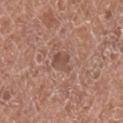<record>
<biopsy_status>not biopsied; imaged during a skin examination</biopsy_status>
<image>
  <source>total-body photography crop</source>
  <field_of_view_mm>15</field_of_view_mm>
</image>
<lighting>white-light</lighting>
<patient>
  <sex>female</sex>
  <age_approx>50</age_approx>
</patient>
<site>right lower leg</site>
<lesion_size>
  <long_diameter_mm_approx>2.5</long_diameter_mm_approx>
</lesion_size>
</record>